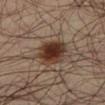{
  "biopsy_status": "not biopsied; imaged during a skin examination",
  "automated_metrics": {
    "eccentricity": 0.65,
    "border_irregularity_0_10": 2.5,
    "color_variation_0_10": 5.5
  },
  "image": {
    "source": "total-body photography crop",
    "field_of_view_mm": 15
  },
  "site": "left lower leg",
  "patient": {
    "sex": "male",
    "age_approx": 35
  },
  "lesion_size": {
    "long_diameter_mm_approx": 4.5
  },
  "lighting": "cross-polarized"
}